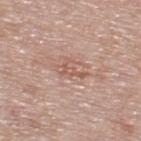* biopsy status: total-body-photography surveillance lesion; no biopsy
* lesion diameter: ≈3 mm
* patient: male, in their mid-50s
* acquisition: total-body-photography crop, ~15 mm field of view
* illumination: white-light illumination
* TBP lesion metrics: a lesion area of about 3.5 mm², an eccentricity of roughly 0.85, and two-axis asymmetry of about 0.55; a lesion color around L≈57 a*≈21 b*≈27 in CIELAB and roughly 8 lightness units darker than nearby skin
* anatomic site: the upper back